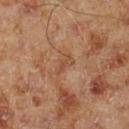  biopsy_status: not biopsied; imaged during a skin examination
  lighting: cross-polarized
  image:
    source: total-body photography crop
    field_of_view_mm: 15
  patient:
    sex: male
    age_approx: 70
  site: left lower leg
  lesion_size:
    long_diameter_mm_approx: 2.5